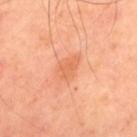Captured during whole-body skin photography for melanoma surveillance; the lesion was not biopsied.
A male patient roughly 50 years of age.
A lesion tile, about 15 mm wide, cut from a 3D total-body photograph.
The lesion's longest dimension is about 3.5 mm.
Located on the back.